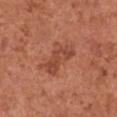Recorded during total-body skin imaging; not selected for excision or biopsy.
Cropped from a total-body skin-imaging series; the visible field is about 15 mm.
From the chest.
The patient is a female aged approximately 50.